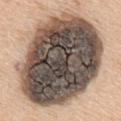Clinical impression:
The lesion was photographed on a routine skin check and not biopsied; there is no pathology result.
Clinical summary:
A female subject, aged 63–67. Captured under white-light illumination. Approximately 12.5 mm at its widest. The lesion is located on the mid back. Automated image analysis of the tile measured a mean CIELAB color near L≈48 a*≈11 b*≈21 and about 26 CIELAB-L* units darker than the surrounding skin. And it measured border irregularity of about 1.5 on a 0–10 scale, internal color variation of about 10 on a 0–10 scale, and peripheral color asymmetry of about 4. The analysis additionally found a classifier nevus-likeness of about 0/100 and a detector confidence of about 85 out of 100 that the crop contains a lesion. A roughly 15 mm field-of-view crop from a total-body skin photograph.